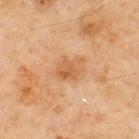Q: Was a biopsy performed?
A: no biopsy performed (imaged during a skin exam)
Q: What is the lesion's diameter?
A: about 3 mm
Q: Where on the body is the lesion?
A: the back
Q: What kind of image is this?
A: 15 mm crop, total-body photography
Q: What did automated image analysis measure?
A: an area of roughly 6.5 mm² and an outline eccentricity of about 0.65 (0 = round, 1 = elongated); an automated nevus-likeness rating near 0 out of 100 and lesion-presence confidence of about 100/100
Q: Illumination type?
A: cross-polarized illumination
Q: Who is the patient?
A: male, roughly 45 years of age